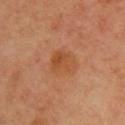Image and clinical context: Imaged with cross-polarized lighting. Measured at roughly 3.5 mm in maximum diameter. Located on the left upper arm. The patient is a female aged approximately 60. Automated tile analysis of the lesion measured a footprint of about 8 mm² and a shape eccentricity near 0.6. The software also gave a mean CIELAB color near L≈52 a*≈26 b*≈39, about 7 CIELAB-L* units darker than the surrounding skin, and a normalized border contrast of about 6. The software also gave a border-irregularity index near 2/10 and a within-lesion color-variation index near 4/10. The analysis additionally found a detector confidence of about 100 out of 100 that the crop contains a lesion. A 15 mm close-up tile from a total-body photography series done for melanoma screening.